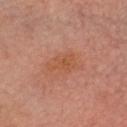biopsy_status: not biopsied; imaged during a skin examination
automated_metrics:
  cielab_L: 46
  cielab_a: 23
  cielab_b: 31
  vs_skin_darker_L: 5.0
lesion_size:
  long_diameter_mm_approx: 3.5
lighting: cross-polarized
image:
  source: total-body photography crop
  field_of_view_mm: 15
patient:
  sex: male
  age_approx: 60
site: head or neck